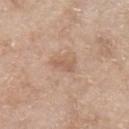biopsy_status: not biopsied; imaged during a skin examination
image:
  source: total-body photography crop
  field_of_view_mm: 15
automated_metrics:
  border_irregularity_0_10: 3.5
  color_variation_0_10: 1.0
  peripheral_color_asymmetry: 0.5
patient:
  sex: male
  age_approx: 65
site: chest